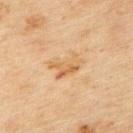Impression:
Recorded during total-body skin imaging; not selected for excision or biopsy.
Context:
Imaged with cross-polarized lighting. Measured at roughly 4 mm in maximum diameter. Cropped from a whole-body photographic skin survey; the tile spans about 15 mm. A male subject, aged 43–47. The lesion is located on the upper back.